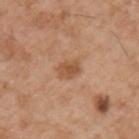follow-up = imaged on a skin check; not biopsied
subject = male, roughly 55 years of age
automated lesion analysis = an automated nevus-likeness rating near 55 out of 100 and a detector confidence of about 100 out of 100 that the crop contains a lesion
site = the left upper arm
lesion diameter = ≈3 mm
acquisition = total-body-photography crop, ~15 mm field of view
tile lighting = white-light